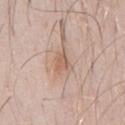Q: Was a biopsy performed?
A: catalogued during a skin exam; not biopsied
Q: What did automated image analysis measure?
A: a lesion area of about 4 mm², an eccentricity of roughly 0.7, and a shape-asymmetry score of about 0.3 (0 = symmetric); a lesion color around L≈60 a*≈19 b*≈28 in CIELAB, a lesion–skin lightness drop of about 9, and a lesion-to-skin contrast of about 6 (normalized; higher = more distinct)
Q: Patient demographics?
A: male, in their 30s
Q: What is the imaging modality?
A: total-body-photography crop, ~15 mm field of view
Q: Lesion location?
A: the chest
Q: How large is the lesion?
A: about 2.5 mm